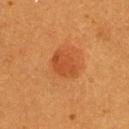image-analysis metrics — a color-variation rating of about 2/10 and peripheral color asymmetry of about 0.5
lesion size — about 3.5 mm
illumination — cross-polarized
imaging modality — total-body-photography crop, ~15 mm field of view
patient — female, about 55 years old
anatomic site — the upper back